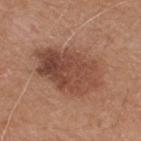• biopsy status — catalogued during a skin exam; not biopsied
• lesion diameter — about 8 mm
• image — ~15 mm crop, total-body skin-cancer survey
• anatomic site — the back
• lighting — white-light
• patient — male, aged 63 to 67